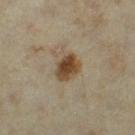Clinical impression:
The lesion was photographed on a routine skin check and not biopsied; there is no pathology result.
Clinical summary:
Approximately 3 mm at its widest. The lesion is on the left thigh. The total-body-photography lesion software estimated roughly 13 lightness units darker than nearby skin and a normalized border contrast of about 11.5. Captured under cross-polarized illumination. This image is a 15 mm lesion crop taken from a total-body photograph. A female patient roughly 35 years of age.